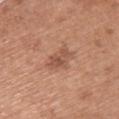Q: Is there a histopathology result?
A: total-body-photography surveillance lesion; no biopsy
Q: Lesion location?
A: the back
Q: Who is the patient?
A: female, aged 58 to 62
Q: How was this image acquired?
A: total-body-photography crop, ~15 mm field of view